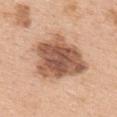workup: catalogued during a skin exam; not biopsied
TBP lesion metrics: a footprint of about 29 mm², an outline eccentricity of about 0.75 (0 = round, 1 = elongated), and a shape-asymmetry score of about 0.25 (0 = symmetric); a lesion color around L≈56 a*≈21 b*≈31 in CIELAB, roughly 17 lightness units darker than nearby skin, and a normalized border contrast of about 10.5; a lesion-detection confidence of about 100/100
acquisition: ~15 mm tile from a whole-body skin photo
tile lighting: white-light
patient: female, approximately 65 years of age
diameter: ≈8 mm
anatomic site: the upper back